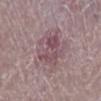Q: Was a biopsy performed?
A: total-body-photography surveillance lesion; no biopsy
Q: What is the anatomic site?
A: the left lower leg
Q: Automated lesion metrics?
A: border irregularity of about 4 on a 0–10 scale; an automated nevus-likeness rating near 15 out of 100
Q: Who is the patient?
A: female, in their mid-60s
Q: How was this image acquired?
A: ~15 mm tile from a whole-body skin photo